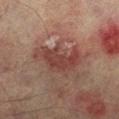Image and clinical context: Located on the right lower leg. Automated tile analysis of the lesion measured an area of roughly 15 mm², an eccentricity of roughly 0.8, and two-axis asymmetry of about 0.35. The analysis additionally found a border-irregularity index near 5.5/10, internal color variation of about 3 on a 0–10 scale, and peripheral color asymmetry of about 1. It also reported a classifier nevus-likeness of about 0/100 and lesion-presence confidence of about 100/100. The tile uses cross-polarized illumination. This image is a 15 mm lesion crop taken from a total-body photograph. A male patient roughly 75 years of age.